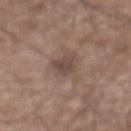– follow-up: no biopsy performed (imaged during a skin exam)
– subject: male, aged 73 to 77
– acquisition: ~15 mm crop, total-body skin-cancer survey
– body site: the abdomen